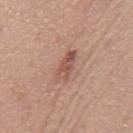Clinical impression: Captured during whole-body skin photography for melanoma surveillance; the lesion was not biopsied. Context: Located on the mid back. The recorded lesion diameter is about 4 mm. A male patient about 55 years old. A 15 mm close-up extracted from a 3D total-body photography capture. The lesion-visualizer software estimated an average lesion color of about L≈53 a*≈22 b*≈26 (CIELAB) and about 10 CIELAB-L* units darker than the surrounding skin. The software also gave border irregularity of about 3.5 on a 0–10 scale and internal color variation of about 5 on a 0–10 scale.